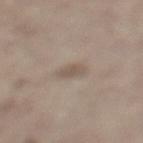Imaged during a routine full-body skin examination; the lesion was not biopsied and no histopathology is available.
A male subject aged around 70.
Longest diameter approximately 3 mm.
This is a white-light tile.
From the left lower leg.
A 15 mm close-up extracted from a 3D total-body photography capture.